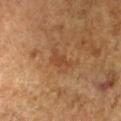Notes:
– follow-up: no biopsy performed (imaged during a skin exam)
– automated metrics: a mean CIELAB color near L≈44 a*≈21 b*≈35 and roughly 6 lightness units darker than nearby skin; a border-irregularity rating of about 5/10, a color-variation rating of about 1/10, and peripheral color asymmetry of about 0; an automated nevus-likeness rating near 5 out of 100 and lesion-presence confidence of about 100/100
– acquisition: ~15 mm crop, total-body skin-cancer survey
– site: the right forearm
– tile lighting: cross-polarized
– size: ~3 mm (longest diameter)
– subject: female, in their 70s